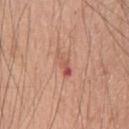biopsy_status: not biopsied; imaged during a skin examination
patient:
  sex: male
  age_approx: 60
image:
  source: total-body photography crop
  field_of_view_mm: 15
lesion_size:
  long_diameter_mm_approx: 3.5
lighting: white-light
automated_metrics:
  cielab_L: 56
  cielab_a: 26
  cielab_b: 30
  vs_skin_darker_L: 9.0
  vs_skin_contrast_norm: 6.0
  border_irregularity_0_10: 5.0
  color_variation_0_10: 4.5
  nevus_likeness_0_100: 0
  lesion_detection_confidence_0_100: 100
site: chest